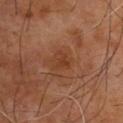Background: About 2.5 mm across. From the back. A male patient, aged around 55. This image is a 15 mm lesion crop taken from a total-body photograph.